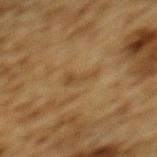follow-up: imaged on a skin check; not biopsied
anatomic site: the back
illumination: cross-polarized illumination
patient: male, aged around 85 or older
image source: 15 mm crop, total-body photography
lesion size: ~3.5 mm (longest diameter)